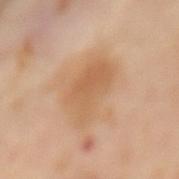biopsy_status: not biopsied; imaged during a skin examination
image:
  source: total-body photography crop
  field_of_view_mm: 15
site: mid back
patient:
  sex: female
  age_approx: 55
automated_metrics:
  cielab_L: 61
  cielab_a: 20
  cielab_b: 36
  vs_skin_darker_L: 8.0
  vs_skin_contrast_norm: 6.0
  nevus_likeness_0_100: 30
lesion_size:
  long_diameter_mm_approx: 6.5
lighting: cross-polarized